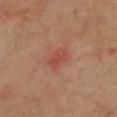Captured during whole-body skin photography for melanoma surveillance; the lesion was not biopsied.